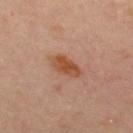workup: catalogued during a skin exam; not biopsied | image: total-body-photography crop, ~15 mm field of view | site: the chest | patient: female, about 50 years old | lighting: cross-polarized | lesion size: ~4 mm (longest diameter).